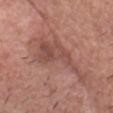biopsy status: total-body-photography surveillance lesion; no biopsy | automated metrics: a color-variation rating of about 4/10 and peripheral color asymmetry of about 1.5; a classifier nevus-likeness of about 0/100 | body site: the head or neck | patient: male, approximately 55 years of age | image source: total-body-photography crop, ~15 mm field of view | lighting: white-light | lesion size: ≈8.5 mm.